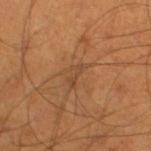Assessment:
Imaged during a routine full-body skin examination; the lesion was not biopsied and no histopathology is available.
Acquisition and patient details:
On the leg. Automated image analysis of the tile measured a within-lesion color-variation index near 0/10 and radial color variation of about 0. A male subject, in their mid- to late 50s. The lesion's longest dimension is about 3 mm. A 15 mm crop from a total-body photograph taken for skin-cancer surveillance.